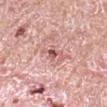Imaged during a routine full-body skin examination; the lesion was not biopsied and no histopathology is available.
A male patient in their mid-60s.
A region of skin cropped from a whole-body photographic capture, roughly 15 mm wide.
The lesion is located on the right upper arm.
The recorded lesion diameter is about 3 mm.